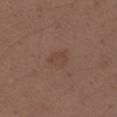Assessment: Imaged during a routine full-body skin examination; the lesion was not biopsied and no histopathology is available. Clinical summary: The subject is a male approximately 40 years of age. The total-body-photography lesion software estimated an area of roughly 4.5 mm², an outline eccentricity of about 0.6 (0 = round, 1 = elongated), and a symmetry-axis asymmetry near 0.3. It also reported a lesion color around L≈43 a*≈17 b*≈26 in CIELAB and a lesion-to-skin contrast of about 4.5 (normalized; higher = more distinct). And it measured an automated nevus-likeness rating near 0 out of 100 and a detector confidence of about 100 out of 100 that the crop contains a lesion. A roughly 15 mm field-of-view crop from a total-body skin photograph. The lesion is on the left upper arm.